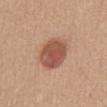Q: What are the patient's age and sex?
A: female, approximately 55 years of age
Q: Lesion size?
A: ~4.5 mm (longest diameter)
Q: How was the tile lit?
A: white-light illumination
Q: What kind of image is this?
A: 15 mm crop, total-body photography
Q: Where on the body is the lesion?
A: the abdomen